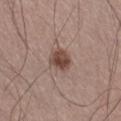Automated tile analysis of the lesion measured a lesion color around L≈46 a*≈18 b*≈23 in CIELAB, a lesion–skin lightness drop of about 13, and a normalized lesion–skin contrast near 9.5. The software also gave an automated nevus-likeness rating near 95 out of 100 and lesion-presence confidence of about 100/100.
A male subject roughly 55 years of age.
A 15 mm crop from a total-body photograph taken for skin-cancer surveillance.
Approximately 3 mm at its widest.
The lesion is on the leg.